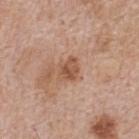The lesion was photographed on a routine skin check and not biopsied; there is no pathology result.